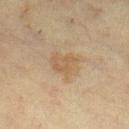Q: What is the anatomic site?
A: the right lower leg
Q: Who is the patient?
A: female, aged approximately 55
Q: What lighting was used for the tile?
A: cross-polarized
Q: What is the imaging modality?
A: ~15 mm crop, total-body skin-cancer survey